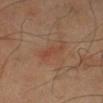This lesion was catalogued during total-body skin photography and was not selected for biopsy.
Longest diameter approximately 3.5 mm.
Located on the right lower leg.
A region of skin cropped from a whole-body photographic capture, roughly 15 mm wide.
The tile uses cross-polarized illumination.
The patient is a male in their 70s.